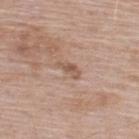Cropped from a whole-body photographic skin survey; the tile spans about 15 mm.
The patient is a male aged 63–67.
Imaged with white-light lighting.
From the upper back.